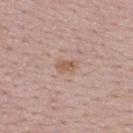Impression: Captured during whole-body skin photography for melanoma surveillance; the lesion was not biopsied. Background: From the back. The recorded lesion diameter is about 2.5 mm. The total-body-photography lesion software estimated a border-irregularity rating of about 3/10, internal color variation of about 1.5 on a 0–10 scale, and radial color variation of about 0.5. The analysis additionally found a nevus-likeness score of about 45/100 and a detector confidence of about 100 out of 100 that the crop contains a lesion. A female subject, about 50 years old. Cropped from a whole-body photographic skin survey; the tile spans about 15 mm. The tile uses white-light illumination.